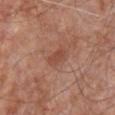notes=no biopsy performed (imaged during a skin exam)
diameter=≈2.5 mm
lighting=white-light
body site=the chest
automated lesion analysis=a lesion area of about 3.5 mm² and an eccentricity of roughly 0.8; a mean CIELAB color near L≈47 a*≈25 b*≈30, a lesion–skin lightness drop of about 7, and a normalized lesion–skin contrast near 6; a border-irregularity rating of about 2.5/10 and a peripheral color-asymmetry measure near 0.5; a lesion-detection confidence of about 100/100
imaging modality=~15 mm tile from a whole-body skin photo
patient=male, in their mid-60s